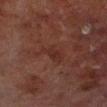Findings:
– workup · no biopsy performed (imaged during a skin exam)